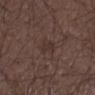Q: Was this lesion biopsied?
A: catalogued during a skin exam; not biopsied
Q: What lighting was used for the tile?
A: white-light
Q: Patient demographics?
A: male, in their 50s
Q: How was this image acquired?
A: ~15 mm crop, total-body skin-cancer survey
Q: Automated lesion metrics?
A: a normalized border contrast of about 5; internal color variation of about 0 on a 0–10 scale and peripheral color asymmetry of about 0; a classifier nevus-likeness of about 5/100 and a lesion-detection confidence of about 100/100
Q: Lesion location?
A: the right forearm
Q: What is the lesion's diameter?
A: ~3 mm (longest diameter)